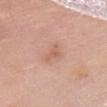biopsy status = catalogued during a skin exam; not biopsied
imaging modality = total-body-photography crop, ~15 mm field of view
lighting = white-light
subject = female, about 60 years old
location = the chest
diameter = about 2.5 mm